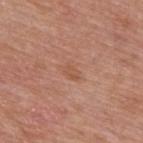Context: About 2.5 mm across. A region of skin cropped from a whole-body photographic capture, roughly 15 mm wide. The tile uses white-light illumination. The patient is a male aged 63 to 67. The lesion is located on the upper back.